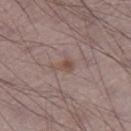– follow-up — no biopsy performed (imaged during a skin exam)
– lesion diameter — about 2.5 mm
– image source — ~15 mm tile from a whole-body skin photo
– patient — male, approximately 70 years of age
– location — the right thigh